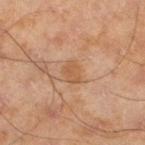Clinical impression:
This lesion was catalogued during total-body skin photography and was not selected for biopsy.
Clinical summary:
A lesion tile, about 15 mm wide, cut from a 3D total-body photograph. Measured at roughly 2.5 mm in maximum diameter. Captured under cross-polarized illumination. The lesion is located on the left lower leg. A male patient, aged approximately 45.